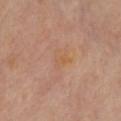follow-up = imaged on a skin check; not biopsied
image = ~15 mm crop, total-body skin-cancer survey
subject = female, approximately 60 years of age
diameter = ~2.5 mm (longest diameter)
body site = the chest
illumination = cross-polarized